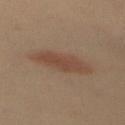image: ~15 mm crop, total-body skin-cancer survey; body site: the mid back; subject: female, aged around 35.